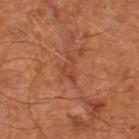Q: Is there a histopathology result?
A: total-body-photography surveillance lesion; no biopsy
Q: Lesion size?
A: ~3 mm (longest diameter)
Q: Who is the patient?
A: male, aged around 65
Q: What is the anatomic site?
A: the leg
Q: Automated lesion metrics?
A: a shape eccentricity near 0.85 and a shape-asymmetry score of about 0.5 (0 = symmetric); roughly 6 lightness units darker than nearby skin; a nevus-likeness score of about 0/100 and a lesion-detection confidence of about 95/100
Q: What lighting was used for the tile?
A: cross-polarized
Q: What is the imaging modality?
A: total-body-photography crop, ~15 mm field of view